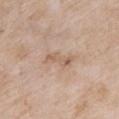{"biopsy_status": "not biopsied; imaged during a skin examination", "automated_metrics": {"cielab_L": 60, "cielab_a": 16, "cielab_b": 30, "vs_skin_darker_L": 8.0, "vs_skin_contrast_norm": 5.5, "nevus_likeness_0_100": 0, "lesion_detection_confidence_0_100": 100}, "patient": {"sex": "female", "age_approx": 75}, "image": {"source": "total-body photography crop", "field_of_view_mm": 15}, "site": "chest"}